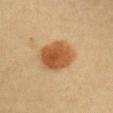workup: total-body-photography surveillance lesion; no biopsy | image source: ~15 mm crop, total-body skin-cancer survey | location: the chest | size: about 5 mm | subject: female, in their 30s | illumination: cross-polarized | automated lesion analysis: a lesion color around L≈49 a*≈20 b*≈37 in CIELAB, roughly 13 lightness units darker than nearby skin, and a normalized lesion–skin contrast near 9.5; a nevus-likeness score of about 100/100.